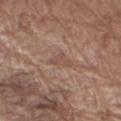Cropped from a total-body skin-imaging series; the visible field is about 15 mm.
The lesion is on the right upper arm.
A male patient aged around 75.